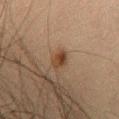Q: Was this lesion biopsied?
A: imaged on a skin check; not biopsied
Q: What are the patient's age and sex?
A: male, aged around 30
Q: Lesion location?
A: the chest
Q: How was the tile lit?
A: cross-polarized
Q: What is the lesion's diameter?
A: ~3 mm (longest diameter)
Q: What kind of image is this?
A: 15 mm crop, total-body photography